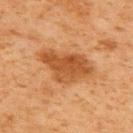follow-up: no biopsy performed (imaged during a skin exam)
automated metrics: an area of roughly 18 mm², a shape eccentricity near 0.8, and a shape-asymmetry score of about 0.35 (0 = symmetric)
location: the upper back
lesion size: about 6.5 mm
tile lighting: cross-polarized illumination
subject: male, aged approximately 60
imaging modality: total-body-photography crop, ~15 mm field of view